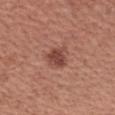The lesion was photographed on a routine skin check and not biopsied; there is no pathology result. A 15 mm crop from a total-body photograph taken for skin-cancer surveillance. Captured under white-light illumination. A male subject about 60 years old. The lesion-visualizer software estimated a mean CIELAB color near L≈45 a*≈25 b*≈26, a lesion–skin lightness drop of about 11, and a lesion-to-skin contrast of about 8 (normalized; higher = more distinct). It also reported a border-irregularity rating of about 3/10, a within-lesion color-variation index near 2/10, and radial color variation of about 1. Located on the left forearm.